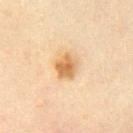Impression: The lesion was photographed on a routine skin check and not biopsied; there is no pathology result. Image and clinical context: A close-up tile cropped from a whole-body skin photograph, about 15 mm across. Approximately 3 mm at its widest. A female patient, approximately 20 years of age. An algorithmic analysis of the crop reported a shape-asymmetry score of about 0.2 (0 = symmetric). It also reported a lesion color around L≈59 a*≈18 b*≈38 in CIELAB and a normalized lesion–skin contrast near 8.5. The analysis additionally found border irregularity of about 2 on a 0–10 scale, a color-variation rating of about 3.5/10, and radial color variation of about 1. It also reported a nevus-likeness score of about 90/100 and a detector confidence of about 100 out of 100 that the crop contains a lesion. Imaged with cross-polarized lighting. From the chest.